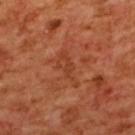Imaged during a routine full-body skin examination; the lesion was not biopsied and no histopathology is available.
On the upper back.
Approximately 4.5 mm at its widest.
A roughly 15 mm field-of-view crop from a total-body skin photograph.
The tile uses cross-polarized illumination.
A female subject about 55 years old.
Automated tile analysis of the lesion measured an area of roughly 5 mm² and an eccentricity of roughly 0.9. And it measured a lesion color around L≈43 a*≈30 b*≈37 in CIELAB, about 6 CIELAB-L* units darker than the surrounding skin, and a lesion-to-skin contrast of about 5 (normalized; higher = more distinct). And it measured a border-irregularity index near 6.5/10, a within-lesion color-variation index near 2.5/10, and peripheral color asymmetry of about 0.5.